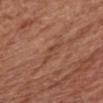Clinical impression: No biopsy was performed on this lesion — it was imaged during a full skin examination and was not determined to be concerning. Acquisition and patient details: This is a white-light tile. The total-body-photography lesion software estimated an outline eccentricity of about 0.9 (0 = round, 1 = elongated) and a symmetry-axis asymmetry near 0.45. The analysis additionally found a lesion color around L≈46 a*≈22 b*≈31 in CIELAB and a normalized lesion–skin contrast near 4.5. Longest diameter approximately 3 mm. Cropped from a total-body skin-imaging series; the visible field is about 15 mm. The lesion is located on the chest. A female subject, about 75 years old.